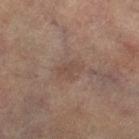Imaged during a routine full-body skin examination; the lesion was not biopsied and no histopathology is available. A 15 mm close-up tile from a total-body photography series done for melanoma screening. Imaged with cross-polarized lighting. A female patient aged approximately 80. The lesion is located on the left leg.